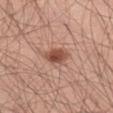follow-up = no biopsy performed (imaged during a skin exam) | image-analysis metrics = an average lesion color of about L≈50 a*≈23 b*≈29 (CIELAB), about 13 CIELAB-L* units darker than the surrounding skin, and a normalized border contrast of about 9; a border-irregularity rating of about 2/10, a color-variation rating of about 4/10, and peripheral color asymmetry of about 1.5 | anatomic site = the left thigh | tile lighting = white-light illumination | patient = male, in their 60s | imaging modality = ~15 mm tile from a whole-body skin photo | lesion diameter = ~3.5 mm (longest diameter).